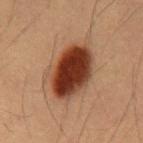Assessment:
The lesion was photographed on a routine skin check and not biopsied; there is no pathology result.
Context:
The subject is a male roughly 55 years of age. The lesion is on the chest. A 15 mm close-up tile from a total-body photography series done for melanoma screening. An algorithmic analysis of the crop reported a mean CIELAB color near L≈33 a*≈22 b*≈28 and roughly 17 lightness units darker than nearby skin. And it measured border irregularity of about 2.5 on a 0–10 scale, a within-lesion color-variation index near 8/10, and peripheral color asymmetry of about 2.5.